On the left upper arm; imaged with white-light lighting; An algorithmic analysis of the crop reported a lesion area of about 22 mm². The software also gave a detector confidence of about 100 out of 100 that the crop contains a lesion; a 15 mm close-up tile from a total-body photography series done for melanoma screening; the subject is a male roughly 55 years of age — 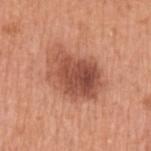Q: What did pathology find?
A: an atypical melanocytic neoplasm — an indeterminate (borderline) lesion